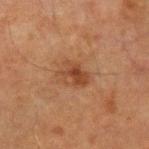biopsy status: no biopsy performed (imaged during a skin exam)
subject: male, roughly 65 years of age
body site: the right forearm
acquisition: ~15 mm tile from a whole-body skin photo